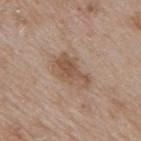Recorded during total-body skin imaging; not selected for excision or biopsy. The subject is a male approximately 65 years of age. The tile uses white-light illumination. The lesion is located on the mid back. A 15 mm crop from a total-body photograph taken for skin-cancer surveillance. The lesion's longest dimension is about 4.5 mm. An algorithmic analysis of the crop reported a mean CIELAB color near L≈52 a*≈18 b*≈29. It also reported a border-irregularity index near 5/10, a within-lesion color-variation index near 2.5/10, and radial color variation of about 0.5. It also reported a classifier nevus-likeness of about 20/100 and a detector confidence of about 100 out of 100 that the crop contains a lesion.